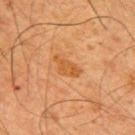  biopsy_status: not biopsied; imaged during a skin examination
  lesion_size:
    long_diameter_mm_approx: 3.5
  image:
    source: total-body photography crop
    field_of_view_mm: 15
  patient:
    sex: male
    age_approx: 65
  lighting: cross-polarized
  site: upper back